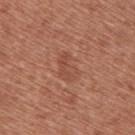biopsy status — total-body-photography surveillance lesion; no biopsy | body site — the back | automated metrics — a lesion area of about 7.5 mm² and a shape-asymmetry score of about 0.2 (0 = symmetric); an automated nevus-likeness rating near 0 out of 100 | patient — female, in their 40s | acquisition — total-body-photography crop, ~15 mm field of view | tile lighting — white-light illumination.